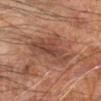Recorded during total-body skin imaging; not selected for excision or biopsy. A subject in their mid- to late 60s. A 15 mm close-up tile from a total-body photography series done for melanoma screening. The lesion is located on the left forearm.